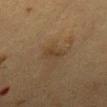Clinical impression: The lesion was photographed on a routine skin check and not biopsied; there is no pathology result. Image and clinical context: An algorithmic analysis of the crop reported a footprint of about 3 mm², an eccentricity of roughly 0.85, and a symmetry-axis asymmetry near 0.3. The analysis additionally found a mean CIELAB color near L≈31 a*≈13 b*≈25 and roughly 5 lightness units darker than nearby skin. And it measured a classifier nevus-likeness of about 5/100. Longest diameter approximately 2.5 mm. The lesion is located on the abdomen. Captured under cross-polarized illumination. The patient is a female aged 48–52. A 15 mm close-up tile from a total-body photography series done for melanoma screening.